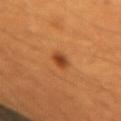lesion_size:
  long_diameter_mm_approx: 2.0
lighting: cross-polarized
site: left forearm
patient:
  sex: female
  age_approx: 40
image:
  source: total-body photography crop
  field_of_view_mm: 15